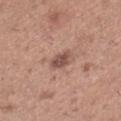biopsy status: imaged on a skin check; not biopsied | body site: the right lower leg | patient: female, approximately 30 years of age | lesion diameter: ~2.5 mm (longest diameter) | imaging modality: total-body-photography crop, ~15 mm field of view.